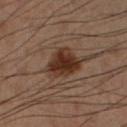Recorded during total-body skin imaging; not selected for excision or biopsy. The lesion is located on the left lower leg. This is a cross-polarized tile. The patient is a male aged 48 to 52. Automated tile analysis of the lesion measured a lesion area of about 12 mm², a shape eccentricity near 0.75, and two-axis asymmetry of about 0.3. The software also gave a lesion–skin lightness drop of about 9 and a normalized border contrast of about 10.5. It also reported border irregularity of about 3 on a 0–10 scale, a within-lesion color-variation index near 3.5/10, and peripheral color asymmetry of about 1. A close-up tile cropped from a whole-body skin photograph, about 15 mm across.